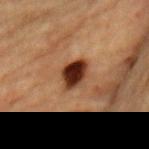imaging modality = ~15 mm tile from a whole-body skin photo
size = ≈3.5 mm
location = the chest
TBP lesion metrics = a lesion area of about 7.5 mm², an eccentricity of roughly 0.65, and a symmetry-axis asymmetry near 0.2; internal color variation of about 6 on a 0–10 scale
lighting = cross-polarized
patient = male, about 85 years old or older